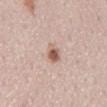No biopsy was performed on this lesion — it was imaged during a full skin examination and was not determined to be concerning. About 3 mm across. The lesion is on the mid back. A lesion tile, about 15 mm wide, cut from a 3D total-body photograph. The tile uses white-light illumination. The total-body-photography lesion software estimated a lesion area of about 5 mm², an outline eccentricity of about 0.85 (0 = round, 1 = elongated), and a symmetry-axis asymmetry near 0.25. The analysis additionally found border irregularity of about 2.5 on a 0–10 scale and peripheral color asymmetry of about 2. A female patient, aged 48 to 52.